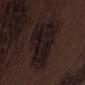Automated image analysis of the tile measured a border-irregularity index near 4/10 and peripheral color asymmetry of about 1. The lesion is located on the right thigh. A male patient, aged approximately 70. Longest diameter approximately 8.5 mm. A lesion tile, about 15 mm wide, cut from a 3D total-body photograph.